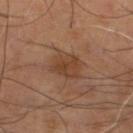Acquisition and patient details: A lesion tile, about 15 mm wide, cut from a 3D total-body photograph. The lesion's longest dimension is about 4 mm. The lesion is on the leg. Captured under cross-polarized illumination. The patient is a male aged around 70.